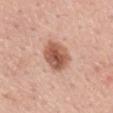biopsy status = no biopsy performed (imaged during a skin exam)
image source = total-body-photography crop, ~15 mm field of view
location = the back
lesion size = ~4 mm (longest diameter)
patient = male, aged approximately 55